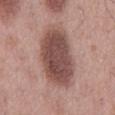Q: Was this lesion biopsied?
A: no biopsy performed (imaged during a skin exam)
Q: How was the tile lit?
A: white-light illumination
Q: Patient demographics?
A: male, aged 53–57
Q: What kind of image is this?
A: 15 mm crop, total-body photography
Q: Lesion location?
A: the mid back